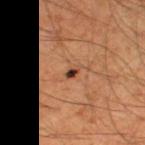| feature | finding |
|---|---|
| workup | catalogued during a skin exam; not biopsied |
| site | the left thigh |
| lesion size | ≈1.5 mm |
| imaging modality | ~15 mm crop, total-body skin-cancer survey |
| subject | male, aged 43–47 |
| lighting | cross-polarized |